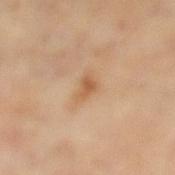Q: Is there a histopathology result?
A: catalogued during a skin exam; not biopsied
Q: What is the imaging modality?
A: ~15 mm tile from a whole-body skin photo
Q: Where on the body is the lesion?
A: the leg
Q: What is the lesion's diameter?
A: ~2.5 mm (longest diameter)
Q: What did automated image analysis measure?
A: about 9 CIELAB-L* units darker than the surrounding skin and a normalized lesion–skin contrast near 6.5; border irregularity of about 3.5 on a 0–10 scale and a within-lesion color-variation index near 1/10
Q: How was the tile lit?
A: cross-polarized illumination
Q: Who is the patient?
A: female, aged approximately 60